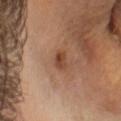A 15 mm close-up extracted from a 3D total-body photography capture. The patient is a male about 45 years old. This is a cross-polarized tile. From the head or neck.